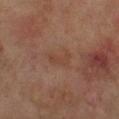follow-up = no biopsy performed (imaged during a skin exam)
tile lighting = cross-polarized
acquisition = 15 mm crop, total-body photography
location = the right lower leg
lesion diameter = ~3 mm (longest diameter)
subject = male, approximately 70 years of age